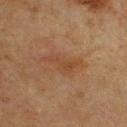<lesion>
<biopsy_status>not biopsied; imaged during a skin examination</biopsy_status>
<image>
  <source>total-body photography crop</source>
  <field_of_view_mm>15</field_of_view_mm>
</image>
<lesion_size>
  <long_diameter_mm_approx>5.0</long_diameter_mm_approx>
</lesion_size>
<patient>
  <sex>male</sex>
  <age_approx>75</age_approx>
</patient>
<site>chest</site>
</lesion>